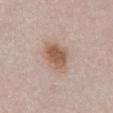<case>
  <biopsy_status>not biopsied; imaged during a skin examination</biopsy_status>
  <patient>
    <sex>female</sex>
    <age_approx>50</age_approx>
  </patient>
  <site>abdomen</site>
  <automated_metrics>
    <cielab_L>57</cielab_L>
    <cielab_a>18</cielab_a>
    <cielab_b>28</cielab_b>
    <vs_skin_darker_L>11.0</vs_skin_darker_L>
    <vs_skin_contrast_norm>8.0</vs_skin_contrast_norm>
    <color_variation_0_10>4.5</color_variation_0_10>
    <nevus_likeness_0_100>100</nevus_likeness_0_100>
    <lesion_detection_confidence_0_100>100</lesion_detection_confidence_0_100>
  </automated_metrics>
  <lighting>white-light</lighting>
  <image>
    <source>total-body photography crop</source>
    <field_of_view_mm>15</field_of_view_mm>
  </image>
  <lesion_size>
    <long_diameter_mm_approx>4.0</long_diameter_mm_approx>
  </lesion_size>
</case>